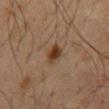follow-up — total-body-photography surveillance lesion; no biopsy | tile lighting — cross-polarized | anatomic site — the mid back | patient — male, roughly 45 years of age | size — about 3 mm | image source — total-body-photography crop, ~15 mm field of view | automated metrics — a lesion area of about 4.5 mm², a shape eccentricity near 0.75, and a shape-asymmetry score of about 0.2 (0 = symmetric); an average lesion color of about L≈36 a*≈18 b*≈29 (CIELAB), roughly 11 lightness units darker than nearby skin, and a lesion-to-skin contrast of about 10 (normalized; higher = more distinct); a border-irregularity index near 2/10 and a peripheral color-asymmetry measure near 1.5.